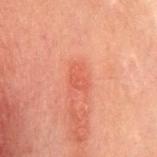workup: total-body-photography surveillance lesion; no biopsy
imaging modality: ~15 mm tile from a whole-body skin photo
diameter: ≈2.5 mm
subject: female, approximately 20 years of age
automated lesion analysis: a lesion–skin lightness drop of about 6 and a normalized border contrast of about 5
tile lighting: cross-polarized
site: the chest